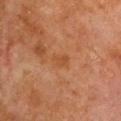This lesion was catalogued during total-body skin photography and was not selected for biopsy.
The lesion is located on the upper back.
The patient is a female aged 78–82.
This image is a 15 mm lesion crop taken from a total-body photograph.